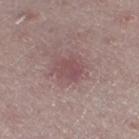Captured during whole-body skin photography for melanoma surveillance; the lesion was not biopsied. The lesion is on the left lower leg. The subject is a female in their mid-40s. The total-body-photography lesion software estimated a border-irregularity index near 2/10, a color-variation rating of about 3/10, and radial color variation of about 1. It also reported a classifier nevus-likeness of about 5/100. A 15 mm close-up extracted from a 3D total-body photography capture. Captured under white-light illumination.